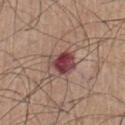Notes:
* workup · no biopsy performed (imaged during a skin exam)
* illumination · white-light illumination
* diameter · ~3.5 mm (longest diameter)
* TBP lesion metrics · an average lesion color of about L≈42 a*≈26 b*≈19 (CIELAB) and a lesion-to-skin contrast of about 12 (normalized; higher = more distinct); border irregularity of about 2.5 on a 0–10 scale, a color-variation rating of about 6.5/10, and radial color variation of about 2
* subject · male, aged approximately 55
* site · the left lower leg
* image · ~15 mm tile from a whole-body skin photo